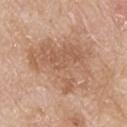Part of a total-body skin-imaging series; this lesion was reviewed on a skin check and was not flagged for biopsy. The subject is a male aged 78–82. Cropped from a whole-body photographic skin survey; the tile spans about 15 mm. On the upper back.